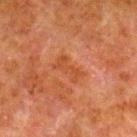The lesion was tiled from a total-body skin photograph and was not biopsied. An algorithmic analysis of the crop reported a lesion area of about 6 mm², a shape eccentricity near 0.85, and two-axis asymmetry of about 0.3. The software also gave a lesion color around L≈39 a*≈25 b*≈33 in CIELAB and a normalized border contrast of about 6. The software also gave a border-irregularity index near 4/10 and a peripheral color-asymmetry measure near 0.5. From the right lower leg. A male subject, in their 80s. This is a cross-polarized tile. Cropped from a total-body skin-imaging series; the visible field is about 15 mm. The recorded lesion diameter is about 3.5 mm.